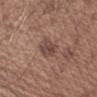| key | value |
|---|---|
| automated lesion analysis | a lesion area of about 5 mm², an outline eccentricity of about 0.7 (0 = round, 1 = elongated), and a shape-asymmetry score of about 0.25 (0 = symmetric); a border-irregularity rating of about 2.5/10, a color-variation rating of about 3/10, and peripheral color asymmetry of about 1 |
| location | the left upper arm |
| subject | male, in their 50s |
| image | 15 mm crop, total-body photography |
| size | about 3 mm |